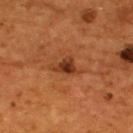Case summary:
– biopsy status — no biopsy performed (imaged during a skin exam)
– subject — male, about 55 years old
– acquisition — 15 mm crop, total-body photography
– lighting — cross-polarized
– lesion size — ≈3 mm
– site — the upper back